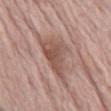No biopsy was performed on this lesion — it was imaged during a full skin examination and was not determined to be concerning.